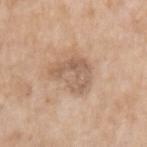Impression: This lesion was catalogued during total-body skin photography and was not selected for biopsy. Clinical summary: The subject is a female approximately 75 years of age. The tile uses white-light illumination. A roughly 15 mm field-of-view crop from a total-body skin photograph. The lesion-visualizer software estimated a footprint of about 10 mm². The analysis additionally found a mean CIELAB color near L≈59 a*≈17 b*≈30 and a normalized lesion–skin contrast near 6. The software also gave a border-irregularity rating of about 7/10. The lesion is on the left upper arm.